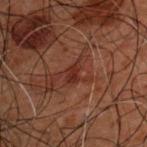imaging modality = ~15 mm tile from a whole-body skin photo; patient = male, about 50 years old; anatomic site = the head or neck.